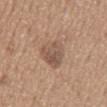Impression:
Part of a total-body skin-imaging series; this lesion was reviewed on a skin check and was not flagged for biopsy.
Background:
This is a white-light tile. The subject is a male aged around 65. A 15 mm close-up extracted from a 3D total-body photography capture. Approximately 4.5 mm at its widest. Located on the mid back. The total-body-photography lesion software estimated an outline eccentricity of about 0.75 (0 = round, 1 = elongated) and a shape-asymmetry score of about 0.3 (0 = symmetric). It also reported about 10 CIELAB-L* units darker than the surrounding skin and a normalized lesion–skin contrast near 7. The analysis additionally found a border-irregularity index near 3/10, a within-lesion color-variation index near 4/10, and peripheral color asymmetry of about 1.5.